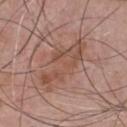biopsy status: imaged on a skin check; not biopsied | subject: male, aged 53–57 | acquisition: ~15 mm crop, total-body skin-cancer survey | automated metrics: a footprint of about 14 mm², a shape eccentricity near 0.95, and a shape-asymmetry score of about 0.4 (0 = symmetric); a lesion color around L≈50 a*≈21 b*≈27 in CIELAB, roughly 8 lightness units darker than nearby skin, and a normalized border contrast of about 6.5; internal color variation of about 4.5 on a 0–10 scale and a peripheral color-asymmetry measure near 1.5; an automated nevus-likeness rating near 15 out of 100 and lesion-presence confidence of about 95/100 | site: the chest | lesion size: ≈7.5 mm.